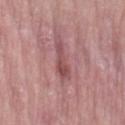Imaged during a routine full-body skin examination; the lesion was not biopsied and no histopathology is available. The total-body-photography lesion software estimated an area of roughly 5.5 mm², an outline eccentricity of about 0.95 (0 = round, 1 = elongated), and a symmetry-axis asymmetry near 0.45. It also reported a border-irregularity index near 5/10, a color-variation rating of about 1.5/10, and a peripheral color-asymmetry measure near 0.5. It also reported a lesion-detection confidence of about 80/100. From the leg. A female patient aged 63 to 67. Longest diameter approximately 4 mm. A lesion tile, about 15 mm wide, cut from a 3D total-body photograph. The tile uses white-light illumination.